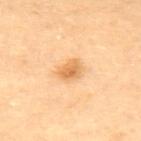No biopsy was performed on this lesion — it was imaged during a full skin examination and was not determined to be concerning.
The lesion is on the upper back.
The tile uses cross-polarized illumination.
Measured at roughly 3 mm in maximum diameter.
A 15 mm close-up extracted from a 3D total-body photography capture.
The patient is a female aged 63–67.
Automated image analysis of the tile measured a footprint of about 5 mm², an outline eccentricity of about 0.65 (0 = round, 1 = elongated), and two-axis asymmetry of about 0.25. The software also gave a mean CIELAB color near L≈70 a*≈23 b*≈46, about 11 CIELAB-L* units darker than the surrounding skin, and a normalized border contrast of about 7.5. And it measured a within-lesion color-variation index near 3.5/10 and radial color variation of about 1. The software also gave an automated nevus-likeness rating near 90 out of 100 and a lesion-detection confidence of about 100/100.